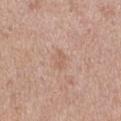The lesion was photographed on a routine skin check and not biopsied; there is no pathology result. A region of skin cropped from a whole-body photographic capture, roughly 15 mm wide. Approximately 3 mm at its widest. A female patient, aged 38–42. From the left thigh.